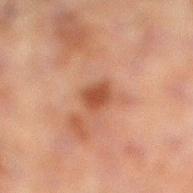This lesion was catalogued during total-body skin photography and was not selected for biopsy. The recorded lesion diameter is about 2.5 mm. Captured under cross-polarized illumination. A roughly 15 mm field-of-view crop from a total-body skin photograph. On the left leg. The lesion-visualizer software estimated a lesion area of about 4.5 mm², an outline eccentricity of about 0.6 (0 = round, 1 = elongated), and a shape-asymmetry score of about 0.25 (0 = symmetric). And it measured a mean CIELAB color near L≈37 a*≈21 b*≈28, roughly 9 lightness units darker than nearby skin, and a normalized border contrast of about 8. The analysis additionally found a border-irregularity rating of about 2/10, a color-variation rating of about 2/10, and peripheral color asymmetry of about 0.5. The analysis additionally found a classifier nevus-likeness of about 85/100. A male subject approximately 60 years of age.